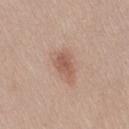<lesion>
  <biopsy_status>not biopsied; imaged during a skin examination</biopsy_status>
  <lesion_size>
    <long_diameter_mm_approx>3.0</long_diameter_mm_approx>
  </lesion_size>
  <lighting>white-light</lighting>
  <patient>
    <sex>female</sex>
    <age_approx>25</age_approx>
  </patient>
  <image>
    <source>total-body photography crop</source>
    <field_of_view_mm>15</field_of_view_mm>
  </image>
  <site>right thigh</site>
</lesion>